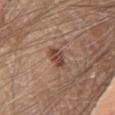Part of a total-body skin-imaging series; this lesion was reviewed on a skin check and was not flagged for biopsy. The total-body-photography lesion software estimated a border-irregularity rating of about 2/10, internal color variation of about 3.5 on a 0–10 scale, and peripheral color asymmetry of about 1. It also reported a classifier nevus-likeness of about 55/100. On the chest. A roughly 15 mm field-of-view crop from a total-body skin photograph. A male patient aged 78 to 82.